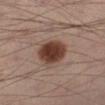The lesion was tiled from a total-body skin photograph and was not biopsied. A 15 mm close-up tile from a total-body photography series done for melanoma screening. The recorded lesion diameter is about 4 mm. This is a cross-polarized tile. The lesion is located on the left lower leg. A male subject, about 40 years old.